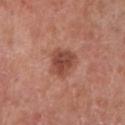Assessment:
Captured during whole-body skin photography for melanoma surveillance; the lesion was not biopsied.
Clinical summary:
Longest diameter approximately 3.5 mm. A male patient, approximately 80 years of age. A roughly 15 mm field-of-view crop from a total-body skin photograph. Located on the right lower leg.